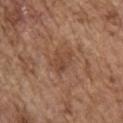biopsy_status: not biopsied; imaged during a skin examination
site: right upper arm
patient:
  sex: male
  age_approx: 75
image:
  source: total-body photography crop
  field_of_view_mm: 15